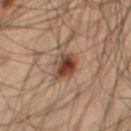This lesion was catalogued during total-body skin photography and was not selected for biopsy.
Cropped from a whole-body photographic skin survey; the tile spans about 15 mm.
From the leg.
A male subject aged 48 to 52.